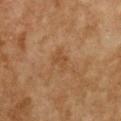The lesion was photographed on a routine skin check and not biopsied; there is no pathology result.